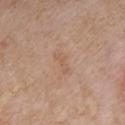notes: imaged on a skin check; not biopsied
site: the chest
patient: male, aged around 75
lesion diameter: about 3 mm
automated metrics: an area of roughly 3 mm², an outline eccentricity of about 0.9 (0 = round, 1 = elongated), and a shape-asymmetry score of about 0.5 (0 = symmetric); a mean CIELAB color near L≈58 a*≈17 b*≈31, roughly 5 lightness units darker than nearby skin, and a normalized border contrast of about 4.5
image source: total-body-photography crop, ~15 mm field of view